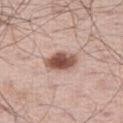The lesion was photographed on a routine skin check and not biopsied; there is no pathology result. This image is a 15 mm lesion crop taken from a total-body photograph. About 4 mm across. From the right thigh. Captured under white-light illumination. A male patient, aged around 60.